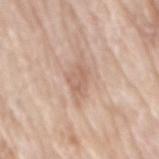This lesion was catalogued during total-body skin photography and was not selected for biopsy. A close-up tile cropped from a whole-body skin photograph, about 15 mm across. From the back. The patient is a male roughly 80 years of age.